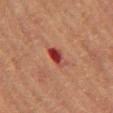<lesion>
  <biopsy_status>not biopsied; imaged during a skin examination</biopsy_status>
  <site>right upper arm</site>
  <patient>
    <sex>female</sex>
    <age_approx>70</age_approx>
  </patient>
  <image>
    <source>total-body photography crop</source>
    <field_of_view_mm>15</field_of_view_mm>
  </image>
  <lesion_size>
    <long_diameter_mm_approx>3.0</long_diameter_mm_approx>
  </lesion_size>
  <lighting>cross-polarized</lighting>
</lesion>